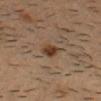follow-up: catalogued during a skin exam; not biopsied
subject: male, aged 33–37
acquisition: total-body-photography crop, ~15 mm field of view
body site: the head or neck